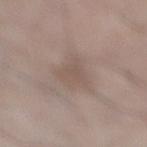The lesion was photographed on a routine skin check and not biopsied; there is no pathology result.
Approximately 4 mm at its widest.
A region of skin cropped from a whole-body photographic capture, roughly 15 mm wide.
The patient is a male aged 53–57.
On the right lower leg.